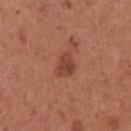Findings:
• follow-up: no biopsy performed (imaged during a skin exam)
• image source: total-body-photography crop, ~15 mm field of view
• diameter: ~3.5 mm (longest diameter)
• body site: the right upper arm
• automated metrics: an area of roughly 5.5 mm², an eccentricity of roughly 0.75, and a symmetry-axis asymmetry near 0.25; a lesion color around L≈44 a*≈26 b*≈29 in CIELAB, about 9 CIELAB-L* units darker than the surrounding skin, and a lesion-to-skin contrast of about 7 (normalized; higher = more distinct); a classifier nevus-likeness of about 50/100
• subject: female, aged around 30